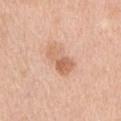Captured during whole-body skin photography for melanoma surveillance; the lesion was not biopsied. Automated image analysis of the tile measured a footprint of about 8.5 mm² and a symmetry-axis asymmetry near 0.2. The software also gave a mean CIELAB color near L≈65 a*≈22 b*≈33, roughly 10 lightness units darker than nearby skin, and a normalized lesion–skin contrast near 6.5. The software also gave border irregularity of about 2.5 on a 0–10 scale, internal color variation of about 7 on a 0–10 scale, and radial color variation of about 2.5. It also reported a nevus-likeness score of about 20/100 and lesion-presence confidence of about 100/100. Located on the left upper arm. The lesion's longest dimension is about 4.5 mm. A close-up tile cropped from a whole-body skin photograph, about 15 mm across. A male patient, roughly 55 years of age. This is a white-light tile.